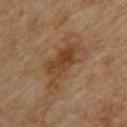follow-up = catalogued during a skin exam; not biopsied | body site = the upper back | image source = ~15 mm tile from a whole-body skin photo | subject = male, about 85 years old or older | lesion size = ≈7.5 mm | tile lighting = cross-polarized.